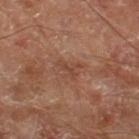Q: Was a biopsy performed?
A: catalogued during a skin exam; not biopsied
Q: Where on the body is the lesion?
A: the leg
Q: What is the imaging modality?
A: 15 mm crop, total-body photography
Q: What is the lesion's diameter?
A: ~3.5 mm (longest diameter)
Q: Who is the patient?
A: male, aged 68–72
Q: What did automated image analysis measure?
A: a footprint of about 5 mm², an eccentricity of roughly 0.75, and a shape-asymmetry score of about 0.4 (0 = symmetric); an average lesion color of about L≈44 a*≈21 b*≈28 (CIELAB), a lesion–skin lightness drop of about 6, and a normalized lesion–skin contrast near 5